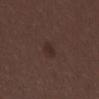  biopsy_status: not biopsied; imaged during a skin examination
  lesion_size:
    long_diameter_mm_approx: 2.5
  site: abdomen
  patient:
    sex: male
    age_approx: 30
  lighting: white-light
  image:
    source: total-body photography crop
    field_of_view_mm: 15